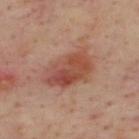| field | value |
|---|---|
| image source | total-body-photography crop, ~15 mm field of view |
| body site | the upper back |
| patient | male, aged 43 to 47 |
| diameter | ≈6 mm |